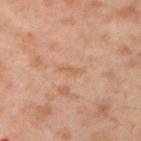Notes:
• location · the right upper arm
• subject · male, aged around 55
• illumination · cross-polarized
• image-analysis metrics · an average lesion color of about L≈60 a*≈21 b*≈35 (CIELAB), a lesion–skin lightness drop of about 6, and a normalized lesion–skin contrast near 5; a border-irregularity rating of about 4.5/10, a color-variation rating of about 0/10, and a peripheral color-asymmetry measure near 0; a nevus-likeness score of about 0/100
• acquisition · total-body-photography crop, ~15 mm field of view
• lesion diameter · about 2.5 mm